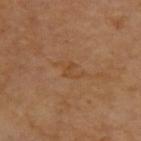Clinical impression:
Part of a total-body skin-imaging series; this lesion was reviewed on a skin check and was not flagged for biopsy.
Background:
From the upper back. The subject is a female roughly 70 years of age. A lesion tile, about 15 mm wide, cut from a 3D total-body photograph.